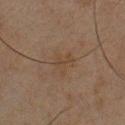{
  "biopsy_status": "not biopsied; imaged during a skin examination",
  "image": {
    "source": "total-body photography crop",
    "field_of_view_mm": 15
  },
  "patient": {
    "sex": "male",
    "age_approx": 45
  },
  "site": "chest"
}